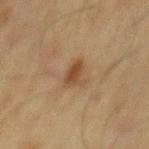  biopsy_status: not biopsied; imaged during a skin examination
  patient:
    sex: male
    age_approx: 70
  lighting: cross-polarized
  image:
    source: total-body photography crop
    field_of_view_mm: 15
  lesion_size:
    long_diameter_mm_approx: 3.0
  site: mid back
  automated_metrics:
    cielab_L: 38
    cielab_a: 16
    cielab_b: 28
    vs_skin_darker_L: 8.0
    vs_skin_contrast_norm: 7.5
    nevus_likeness_0_100: 75
    lesion_detection_confidence_0_100: 100